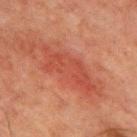notes: no biopsy performed (imaged during a skin exam) | image source: total-body-photography crop, ~15 mm field of view | site: the chest | diameter: ~7.5 mm (longest diameter) | TBP lesion metrics: an average lesion color of about L≈39 a*≈28 b*≈28 (CIELAB), a lesion–skin lightness drop of about 6, and a lesion-to-skin contrast of about 5.5 (normalized; higher = more distinct); a classifier nevus-likeness of about 0/100 and lesion-presence confidence of about 95/100 | lighting: cross-polarized | subject: male, aged around 65.